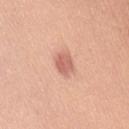Assessment: Part of a total-body skin-imaging series; this lesion was reviewed on a skin check and was not flagged for biopsy. Context: The lesion is on the right thigh. Imaged with white-light lighting. A female subject, approximately 55 years of age. A 15 mm close-up tile from a total-body photography series done for melanoma screening. Measured at roughly 3 mm in maximum diameter.